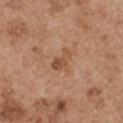Q: Is there a histopathology result?
A: imaged on a skin check; not biopsied
Q: Lesion location?
A: the left upper arm
Q: How was this image acquired?
A: total-body-photography crop, ~15 mm field of view
Q: Who is the patient?
A: male, in their mid-50s
Q: What lighting was used for the tile?
A: white-light illumination
Q: How large is the lesion?
A: ~3 mm (longest diameter)